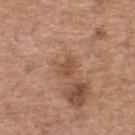Impression: The lesion was tiled from a total-body skin photograph and was not biopsied. Context: This is a white-light tile. The recorded lesion diameter is about 2.5 mm. Located on the upper back. The patient is a female aged around 40. A 15 mm crop from a total-body photograph taken for skin-cancer surveillance. Automated tile analysis of the lesion measured a mean CIELAB color near L≈50 a*≈22 b*≈31 and a normalized border contrast of about 6. And it measured a nevus-likeness score of about 0/100 and a detector confidence of about 100 out of 100 that the crop contains a lesion.